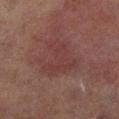Captured during whole-body skin photography for melanoma surveillance; the lesion was not biopsied.
A close-up tile cropped from a whole-body skin photograph, about 15 mm across.
Captured under cross-polarized illumination.
From the leg.
The lesion's longest dimension is about 5 mm.
The patient is a male aged 68 to 72.